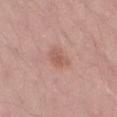biopsy_status: not biopsied; imaged during a skin examination
lighting: white-light
patient:
  sex: male
  age_approx: 50
site: left thigh
image:
  source: total-body photography crop
  field_of_view_mm: 15